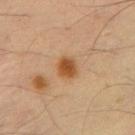workup=total-body-photography surveillance lesion; no biopsy | site=the chest | tile lighting=cross-polarized illumination | subject=male, approximately 55 years of age | lesion diameter=~2.5 mm (longest diameter) | imaging modality=~15 mm tile from a whole-body skin photo.